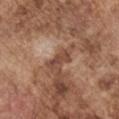Q: Was this lesion biopsied?
A: total-body-photography surveillance lesion; no biopsy
Q: Lesion location?
A: the left upper arm
Q: How was the tile lit?
A: white-light
Q: How was this image acquired?
A: total-body-photography crop, ~15 mm field of view
Q: Lesion size?
A: ≈3 mm
Q: Automated lesion metrics?
A: a border-irregularity index near 5.5/10, a within-lesion color-variation index near 0/10, and peripheral color asymmetry of about 0
Q: Patient demographics?
A: male, aged 73–77